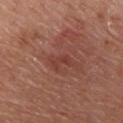Clinical impression: Captured during whole-body skin photography for melanoma surveillance; the lesion was not biopsied. Context: A 15 mm close-up tile from a total-body photography series done for melanoma screening. The lesion is located on the chest. This is a white-light tile. The patient is a male aged 73–77. About 2.5 mm across.